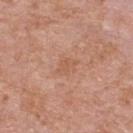This lesion was catalogued during total-body skin photography and was not selected for biopsy. The lesion is located on the back. A roughly 15 mm field-of-view crop from a total-body skin photograph. Longest diameter approximately 2.5 mm. A male subject aged approximately 75.